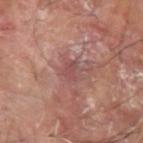From the left forearm. About 3 mm across. A roughly 15 mm field-of-view crop from a total-body skin photograph. The tile uses cross-polarized illumination. A male patient in their mid-60s.